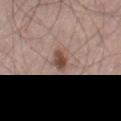workup: imaged on a skin check; not biopsied | automated lesion analysis: an area of roughly 4 mm², an outline eccentricity of about 0.75 (0 = round, 1 = elongated), and two-axis asymmetry of about 0.2; a border-irregularity index near 1.5/10, a within-lesion color-variation index near 4.5/10, and a peripheral color-asymmetry measure near 1.5 | body site: the right thigh | diameter: ~2.5 mm (longest diameter) | patient: male, aged 58–62 | imaging modality: ~15 mm tile from a whole-body skin photo | lighting: white-light illumination.